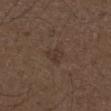Impression: The lesion was tiled from a total-body skin photograph and was not biopsied. Image and clinical context: The tile uses white-light illumination. A male subject, aged 48 to 52. On the leg. Cropped from a whole-body photographic skin survey; the tile spans about 15 mm. Longest diameter approximately 2.5 mm.